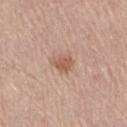Automated tile analysis of the lesion measured a footprint of about 4 mm², an eccentricity of roughly 0.6, and a shape-asymmetry score of about 0.25 (0 = symmetric). It also reported an average lesion color of about L≈57 a*≈21 b*≈29 (CIELAB), about 10 CIELAB-L* units darker than the surrounding skin, and a normalized border contrast of about 7. And it measured a border-irregularity index near 2.5/10, a within-lesion color-variation index near 2/10, and peripheral color asymmetry of about 1. A female subject aged around 70. The lesion is located on the right thigh. Imaged with white-light lighting. A region of skin cropped from a whole-body photographic capture, roughly 15 mm wide.